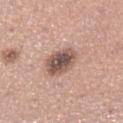Impression: Imaged during a routine full-body skin examination; the lesion was not biopsied and no histopathology is available. Image and clinical context: The lesion is on the leg. A female subject in their 30s. Cropped from a whole-body photographic skin survey; the tile spans about 15 mm. Imaged with white-light lighting. Approximately 4 mm at its widest.